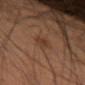Impression: The lesion was photographed on a routine skin check and not biopsied; there is no pathology result. Image and clinical context: From the left forearm. About 3.5 mm across. The tile uses cross-polarized illumination. An algorithmic analysis of the crop reported an area of roughly 3.5 mm² and an eccentricity of roughly 0.9. The software also gave a mean CIELAB color near L≈33 a*≈17 b*≈26, roughly 6 lightness units darker than nearby skin, and a lesion-to-skin contrast of about 6 (normalized; higher = more distinct). It also reported border irregularity of about 4 on a 0–10 scale, a within-lesion color-variation index near 2/10, and a peripheral color-asymmetry measure near 1. The software also gave a nevus-likeness score of about 40/100 and a lesion-detection confidence of about 100/100. Cropped from a total-body skin-imaging series; the visible field is about 15 mm. The subject is a male aged approximately 55.